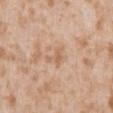{"site": "abdomen", "image": {"source": "total-body photography crop", "field_of_view_mm": 15}, "patient": {"sex": "male", "age_approx": 45}}